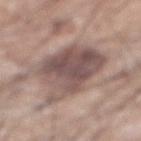The lesion was photographed on a routine skin check and not biopsied; there is no pathology result.
The recorded lesion diameter is about 8 mm.
A roughly 15 mm field-of-view crop from a total-body skin photograph.
From the abdomen.
Automated tile analysis of the lesion measured an area of roughly 30 mm², an outline eccentricity of about 0.7 (0 = round, 1 = elongated), and a symmetry-axis asymmetry near 0.3. The software also gave a mean CIELAB color near L≈51 a*≈16 b*≈20, about 12 CIELAB-L* units darker than the surrounding skin, and a normalized lesion–skin contrast near 9. It also reported a border-irregularity rating of about 3.5/10, a within-lesion color-variation index near 5.5/10, and a peripheral color-asymmetry measure near 2.
The subject is a male in their mid- to late 70s.